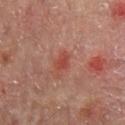acquisition: ~15 mm tile from a whole-body skin photo
tile lighting: cross-polarized illumination
image-analysis metrics: a footprint of about 3.5 mm², an eccentricity of roughly 0.75, and a symmetry-axis asymmetry near 0.3; a mean CIELAB color near L≈45 a*≈29 b*≈29 and a lesion–skin lightness drop of about 7
diameter: ≈2.5 mm
patient: male, about 65 years old
location: the mid back